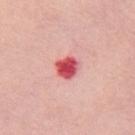follow-up: imaged on a skin check; not biopsied | subject: female, roughly 55 years of age | location: the chest | image source: 15 mm crop, total-body photography.